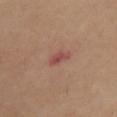acquisition: ~15 mm tile from a whole-body skin photo
TBP lesion metrics: a lesion color around L≈49 a*≈26 b*≈24 in CIELAB, roughly 9 lightness units darker than nearby skin, and a normalized border contrast of about 7; internal color variation of about 2.5 on a 0–10 scale and a peripheral color-asymmetry measure near 1; a classifier nevus-likeness of about 0/100 and lesion-presence confidence of about 100/100
subject: female, in their mid-50s
lighting: cross-polarized
site: the front of the torso
lesion diameter: ≈3 mm